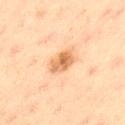Imaged during a routine full-body skin examination; the lesion was not biopsied and no histopathology is available. A roughly 15 mm field-of-view crop from a total-body skin photograph. A male patient, aged 43 to 47. About 3.5 mm across. Automated tile analysis of the lesion measured a footprint of about 5.5 mm², a shape eccentricity near 0.75, and two-axis asymmetry of about 0.25. The analysis additionally found a border-irregularity rating of about 2.5/10, a color-variation rating of about 4.5/10, and a peripheral color-asymmetry measure near 1.5. The lesion is located on the left thigh. The tile uses cross-polarized illumination.